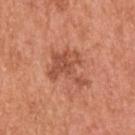anatomic site = the back
image source = total-body-photography crop, ~15 mm field of view
lesion diameter = ~5 mm (longest diameter)
subject = male, about 55 years old